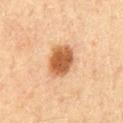No biopsy was performed on this lesion — it was imaged during a full skin examination and was not determined to be concerning.